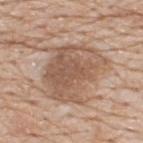Notes:
– notes: no biopsy performed (imaged during a skin exam)
– image: 15 mm crop, total-body photography
– patient: male, roughly 80 years of age
– automated metrics: an area of roughly 27 mm², an eccentricity of roughly 0.65, and a symmetry-axis asymmetry near 0.3; a mean CIELAB color near L≈55 a*≈17 b*≈29, a lesion–skin lightness drop of about 10, and a normalized lesion–skin contrast near 7; a border-irregularity index near 4/10 and radial color variation of about 1.5
– body site: the upper back
– lesion size: about 6.5 mm
– lighting: white-light illumination